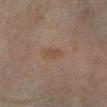The lesion was tiled from a total-body skin photograph and was not biopsied. A female subject, about 60 years old. A 15 mm close-up tile from a total-body photography series done for melanoma screening. The recorded lesion diameter is about 3 mm. Imaged with cross-polarized lighting. Automated image analysis of the tile measured a footprint of about 3 mm² and a shape eccentricity near 0.9. The analysis additionally found a border-irregularity rating of about 2/10, a within-lesion color-variation index near 0.5/10, and radial color variation of about 0. The lesion is located on the left lower leg.